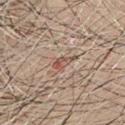Impression:
This lesion was catalogued during total-body skin photography and was not selected for biopsy.
Acquisition and patient details:
This is a cross-polarized tile. The total-body-photography lesion software estimated a shape eccentricity near 0.9 and two-axis asymmetry of about 0.35. The lesion is located on the chest. Cropped from a total-body skin-imaging series; the visible field is about 15 mm. The lesion's longest dimension is about 3 mm. The subject is a male roughly 60 years of age.